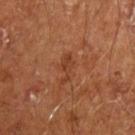Captured during whole-body skin photography for melanoma surveillance; the lesion was not biopsied.
The subject is a male aged 63 to 67.
This is a cross-polarized tile.
A lesion tile, about 15 mm wide, cut from a 3D total-body photograph.
About 3.5 mm across.
On the left upper arm.